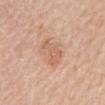Findings:
* workup — no biopsy performed (imaged during a skin exam)
* patient — female, aged 68 to 72
* image — ~15 mm crop, total-body skin-cancer survey
* illumination — white-light
* diameter — ≈3.5 mm
* body site — the mid back